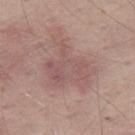Q: Is there a histopathology result?
A: total-body-photography surveillance lesion; no biopsy
Q: Patient demographics?
A: male, aged 63 to 67
Q: How was the tile lit?
A: white-light illumination
Q: Where on the body is the lesion?
A: the right thigh
Q: What is the imaging modality?
A: ~15 mm tile from a whole-body skin photo
Q: What did automated image analysis measure?
A: a lesion area of about 24 mm², a shape eccentricity near 0.6, and two-axis asymmetry of about 0.5; a nevus-likeness score of about 0/100 and a lesion-detection confidence of about 100/100